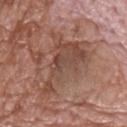Findings:
• location — the back
• subject — male, approximately 70 years of age
• illumination — white-light illumination
• image source — ~15 mm tile from a whole-body skin photo
• automated lesion analysis — a shape-asymmetry score of about 0.6 (0 = symmetric); an average lesion color of about L≈47 a*≈20 b*≈26 (CIELAB), a lesion–skin lightness drop of about 8, and a lesion-to-skin contrast of about 6.5 (normalized; higher = more distinct); a border-irregularity rating of about 8.5/10, internal color variation of about 4.5 on a 0–10 scale, and peripheral color asymmetry of about 1.5
• lesion size — about 8.5 mm